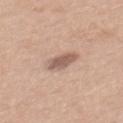tile lighting: white-light | lesion size: about 4 mm | subject: male, approximately 30 years of age | automated lesion analysis: a lesion area of about 6 mm², an eccentricity of roughly 0.9, and two-axis asymmetry of about 0.2; a mean CIELAB color near L≈58 a*≈18 b*≈25 and roughly 12 lightness units darker than nearby skin; a classifier nevus-likeness of about 25/100 and lesion-presence confidence of about 100/100 | site: the mid back | acquisition: total-body-photography crop, ~15 mm field of view.